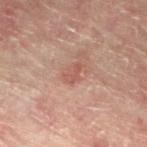Q: Is there a histopathology result?
A: catalogued during a skin exam; not biopsied
Q: Patient demographics?
A: male, aged approximately 60
Q: What kind of image is this?
A: ~15 mm tile from a whole-body skin photo
Q: Automated lesion metrics?
A: roughly 7 lightness units darker than nearby skin and a normalized lesion–skin contrast near 5.5; a border-irregularity index near 5/10, a within-lesion color-variation index near 0/10, and peripheral color asymmetry of about 0; a nevus-likeness score of about 10/100 and a detector confidence of about 100 out of 100 that the crop contains a lesion
Q: What is the anatomic site?
A: the left lower leg
Q: Illumination type?
A: cross-polarized illumination
Q: What is the lesion's diameter?
A: ≈2.5 mm